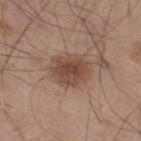Part of a total-body skin-imaging series; this lesion was reviewed on a skin check and was not flagged for biopsy. The lesion is on the right thigh. A 15 mm close-up extracted from a 3D total-body photography capture. A male patient about 45 years old. Longest diameter approximately 4.5 mm. This is a white-light tile. Automated tile analysis of the lesion measured a lesion color around L≈47 a*≈19 b*≈26 in CIELAB and a lesion–skin lightness drop of about 11.